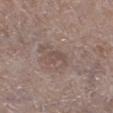Captured during whole-body skin photography for melanoma surveillance; the lesion was not biopsied.
The subject is a male aged approximately 65.
The lesion is located on the left lower leg.
A region of skin cropped from a whole-body photographic capture, roughly 15 mm wide.
Approximately 2.5 mm at its widest.
Automated image analysis of the tile measured a lesion area of about 3.5 mm² and two-axis asymmetry of about 0.35. It also reported an average lesion color of about L≈49 a*≈15 b*≈21 (CIELAB), about 6 CIELAB-L* units darker than the surrounding skin, and a normalized border contrast of about 5. And it measured a peripheral color-asymmetry measure near 0.5. The software also gave a classifier nevus-likeness of about 0/100 and a detector confidence of about 75 out of 100 that the crop contains a lesion.